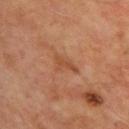Notes:
- biopsy status — no biopsy performed (imaged during a skin exam)
- location — the upper back
- lesion diameter — about 3 mm
- acquisition — ~15 mm crop, total-body skin-cancer survey
- subject — male, aged 63 to 67
- tile lighting — cross-polarized illumination
- automated lesion analysis — an average lesion color of about L≈48 a*≈24 b*≈35 (CIELAB), about 7 CIELAB-L* units darker than the surrounding skin, and a normalized border contrast of about 5.5; a border-irregularity rating of about 5/10, a color-variation rating of about 1/10, and radial color variation of about 0.5; an automated nevus-likeness rating near 0 out of 100 and a detector confidence of about 100 out of 100 that the crop contains a lesion